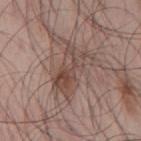workup: catalogued during a skin exam; not biopsied
acquisition: total-body-photography crop, ~15 mm field of view
image-analysis metrics: an outline eccentricity of about 0.85 (0 = round, 1 = elongated) and a shape-asymmetry score of about 0.3 (0 = symmetric); an average lesion color of about L≈47 a*≈17 b*≈23 (CIELAB) and a normalized lesion–skin contrast near 6.5; lesion-presence confidence of about 100/100
anatomic site: the mid back
patient: male, approximately 45 years of age